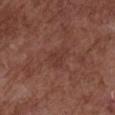– notes: imaged on a skin check; not biopsied
– location: the chest
– diameter: ≈3 mm
– patient: female, aged approximately 80
– image: ~15 mm crop, total-body skin-cancer survey